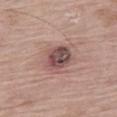lesion size — ~4.5 mm (longest diameter)
image-analysis metrics — a lesion area of about 11 mm², an eccentricity of roughly 0.65, and a shape-asymmetry score of about 0.2 (0 = symmetric); a lesion color around L≈49 a*≈20 b*≈20 in CIELAB, a lesion–skin lightness drop of about 13, and a lesion-to-skin contrast of about 9.5 (normalized; higher = more distinct)
image source — total-body-photography crop, ~15 mm field of view
anatomic site — the right thigh
patient — female, about 75 years old